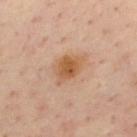{
  "biopsy_status": "not biopsied; imaged during a skin examination",
  "lesion_size": {
    "long_diameter_mm_approx": 4.0
  },
  "patient": {
    "sex": "male",
    "age_approx": 45
  },
  "site": "upper back",
  "image": {
    "source": "total-body photography crop",
    "field_of_view_mm": 15
  }
}